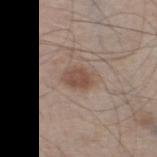Assessment: No biopsy was performed on this lesion — it was imaged during a full skin examination and was not determined to be concerning. Image and clinical context: The lesion is on the left thigh. Cropped from a total-body skin-imaging series; the visible field is about 15 mm. Automated image analysis of the tile measured border irregularity of about 1.5 on a 0–10 scale, a within-lesion color-variation index near 2/10, and a peripheral color-asymmetry measure near 0.5. The subject is a male aged approximately 60. Captured under white-light illumination.